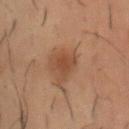patient = male, aged around 30; body site = the abdomen; acquisition = total-body-photography crop, ~15 mm field of view; illumination = cross-polarized; lesion size = ~2.5 mm (longest diameter).